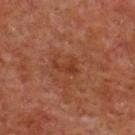Impression:
The lesion was photographed on a routine skin check and not biopsied; there is no pathology result.
Image and clinical context:
The patient is a male about 60 years old. A 15 mm close-up extracted from a 3D total-body photography capture. The lesion is on the chest. Captured under cross-polarized illumination. Measured at roughly 3 mm in maximum diameter. An algorithmic analysis of the crop reported an average lesion color of about L≈34 a*≈25 b*≈30 (CIELAB) and a normalized lesion–skin contrast near 6.